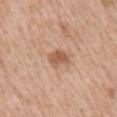  biopsy_status: not biopsied; imaged during a skin examination
  lesion_size:
    long_diameter_mm_approx: 2.5
  image:
    source: total-body photography crop
    field_of_view_mm: 15
  automated_metrics:
    area_mm2_approx: 4.0
    eccentricity: 0.7
    shape_asymmetry: 0.3
    cielab_L: 56
    cielab_a: 22
    cielab_b: 33
    vs_skin_contrast_norm: 7.5
    border_irregularity_0_10: 2.5
    peripheral_color_asymmetry: 0.5
  patient:
    sex: male
    age_approx: 60
  site: right upper arm